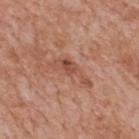Impression:
This lesion was catalogued during total-body skin photography and was not selected for biopsy.
Clinical summary:
A male subject, about 65 years old. A 15 mm close-up extracted from a 3D total-body photography capture. From the mid back.